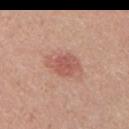biopsy status = no biopsy performed (imaged during a skin exam); automated lesion analysis = a lesion color around L≈56 a*≈26 b*≈27 in CIELAB, about 10 CIELAB-L* units darker than the surrounding skin, and a normalized lesion–skin contrast near 6.5; lesion diameter = ≈3.5 mm; anatomic site = the left upper arm; image source = ~15 mm crop, total-body skin-cancer survey; subject = male, about 25 years old; illumination = white-light illumination.